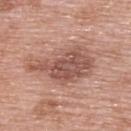Q: Is there a histopathology result?
A: total-body-photography surveillance lesion; no biopsy
Q: What lighting was used for the tile?
A: white-light
Q: What is the lesion's diameter?
A: ~7.5 mm (longest diameter)
Q: Who is the patient?
A: female, aged 58–62
Q: What is the imaging modality?
A: 15 mm crop, total-body photography
Q: What is the anatomic site?
A: the upper back
Q: Automated lesion metrics?
A: a shape eccentricity near 0.85 and two-axis asymmetry of about 0.4; an average lesion color of about L≈53 a*≈22 b*≈26 (CIELAB) and a normalized lesion–skin contrast near 8; a border-irregularity rating of about 4.5/10 and a color-variation rating of about 4/10